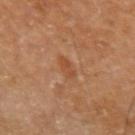The lesion was photographed on a routine skin check and not biopsied; there is no pathology result. The tile uses cross-polarized illumination. The lesion is located on the left upper arm. Cropped from a whole-body photographic skin survey; the tile spans about 15 mm. A male subject, in their 60s. About 2.5 mm across. Automated tile analysis of the lesion measured a shape eccentricity near 0.9 and a symmetry-axis asymmetry near 0.25. It also reported an average lesion color of about L≈46 a*≈23 b*≈35 (CIELAB), a lesion–skin lightness drop of about 7, and a normalized border contrast of about 6. And it measured a border-irregularity index near 3/10, internal color variation of about 0 on a 0–10 scale, and a peripheral color-asymmetry measure near 0. And it measured a nevus-likeness score of about 0/100 and a lesion-detection confidence of about 100/100.